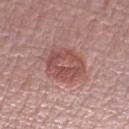Findings:
* biopsy status · catalogued during a skin exam; not biopsied
* patient · male, aged 63 to 67
* site · the left forearm
* acquisition · ~15 mm crop, total-body skin-cancer survey
* illumination · white-light
* automated metrics · a lesion area of about 17 mm², an eccentricity of roughly 0.6, and two-axis asymmetry of about 0.15; internal color variation of about 4.5 on a 0–10 scale and peripheral color asymmetry of about 1.5; an automated nevus-likeness rating near 80 out of 100 and a lesion-detection confidence of about 100/100